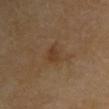Impression:
No biopsy was performed on this lesion — it was imaged during a full skin examination and was not determined to be concerning.
Clinical summary:
A male subject in their 70s. A close-up tile cropped from a whole-body skin photograph, about 15 mm across. On the chest.